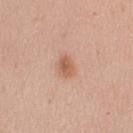size = ≈3 mm | patient = female, approximately 40 years of age | image = 15 mm crop, total-body photography | tile lighting = white-light illumination | location = the left upper arm.